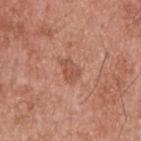Part of a total-body skin-imaging series; this lesion was reviewed on a skin check and was not flagged for biopsy.
A 15 mm close-up tile from a total-body photography series done for melanoma screening.
The tile uses white-light illumination.
The lesion is on the upper back.
A male subject approximately 55 years of age.